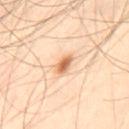  biopsy_status: not biopsied; imaged during a skin examination
  image:
    source: total-body photography crop
    field_of_view_mm: 15
  lesion_size:
    long_diameter_mm_approx: 2.5
  patient:
    sex: male
    age_approx: 45
  automated_metrics:
    cielab_L: 67
    cielab_a: 23
    cielab_b: 38
    vs_skin_darker_L: 15.0
    vs_skin_contrast_norm: 9.0
    border_irregularity_0_10: 2.5
    color_variation_0_10: 3.0
    peripheral_color_asymmetry: 1.0
  lighting: cross-polarized
  site: lower back